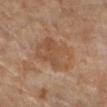Impression:
The lesion was photographed on a routine skin check and not biopsied; there is no pathology result.
Context:
The subject is a female aged 68 to 72. Approximately 5.5 mm at its widest. An algorithmic analysis of the crop reported an area of roughly 17 mm², an outline eccentricity of about 0.8 (0 = round, 1 = elongated), and a shape-asymmetry score of about 0.25 (0 = symmetric). And it measured border irregularity of about 2.5 on a 0–10 scale and a within-lesion color-variation index near 3.5/10. It also reported a lesion-detection confidence of about 100/100. On the leg. Imaged with cross-polarized lighting. A close-up tile cropped from a whole-body skin photograph, about 15 mm across.